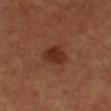  site: leg
  lighting: cross-polarized
  patient:
    sex: male
    age_approx: 50
  image:
    source: total-body photography crop
    field_of_view_mm: 15